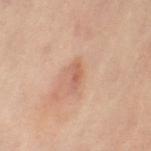biopsy status = imaged on a skin check; not biopsied | body site = the left leg | diameter = ≈2.5 mm | subject = female, aged approximately 60 | lighting = cross-polarized illumination | acquisition = ~15 mm crop, total-body skin-cancer survey.